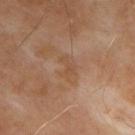| key | value |
|---|---|
| notes | total-body-photography surveillance lesion; no biopsy |
| lighting | cross-polarized |
| imaging modality | 15 mm crop, total-body photography |
| body site | the upper back |
| subject | male, aged around 55 |